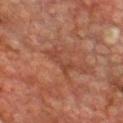notes = imaged on a skin check; not biopsied | location = the chest | subject = male, aged 73 to 77 | acquisition = ~15 mm crop, total-body skin-cancer survey.